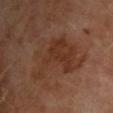{
  "biopsy_status": "not biopsied; imaged during a skin examination",
  "automated_metrics": {
    "area_mm2_approx": 25.0,
    "eccentricity": 0.75,
    "shape_asymmetry": 0.5,
    "cielab_L": 32,
    "cielab_a": 19,
    "cielab_b": 27,
    "vs_skin_darker_L": 6.0,
    "vs_skin_contrast_norm": 6.0,
    "nevus_likeness_0_100": 5,
    "lesion_detection_confidence_0_100": 100
  },
  "image": {
    "source": "total-body photography crop",
    "field_of_view_mm": 15
  },
  "site": "chest",
  "lighting": "cross-polarized",
  "patient": {
    "sex": "male",
    "age_approx": 65
  },
  "lesion_size": {
    "long_diameter_mm_approx": 8.0
  }
}